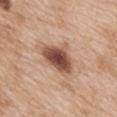The lesion was photographed on a routine skin check and not biopsied; there is no pathology result. A roughly 15 mm field-of-view crop from a total-body skin photograph. Located on the mid back. A female patient, aged 38 to 42.